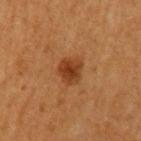Recorded during total-body skin imaging; not selected for excision or biopsy. A 15 mm close-up tile from a total-body photography series done for melanoma screening. The lesion is located on the left upper arm. A male patient, aged 58 to 62. Measured at roughly 3.5 mm in maximum diameter. The lesion-visualizer software estimated an area of roughly 7 mm², an outline eccentricity of about 0.55 (0 = round, 1 = elongated), and two-axis asymmetry of about 0.2. It also reported an average lesion color of about L≈39 a*≈24 b*≈37 (CIELAB) and a lesion–skin lightness drop of about 10. The software also gave a color-variation rating of about 3.5/10 and radial color variation of about 1. It also reported a nevus-likeness score of about 95/100 and a lesion-detection confidence of about 100/100.